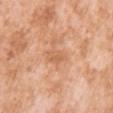Measured at roughly 2.5 mm in maximum diameter. A region of skin cropped from a whole-body photographic capture, roughly 15 mm wide. From the right upper arm. The subject is a female aged approximately 40. This is a white-light tile.